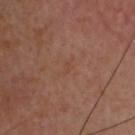- size: ~2 mm (longest diameter)
- anatomic site: the head or neck
- patient: male, aged 63–67
- lighting: cross-polarized
- imaging modality: ~15 mm tile from a whole-body skin photo
- automated lesion analysis: a footprint of about 1.5 mm², an eccentricity of roughly 0.85, and two-axis asymmetry of about 0.4; about 4 CIELAB-L* units darker than the surrounding skin and a lesion-to-skin contrast of about 4 (normalized; higher = more distinct); a border-irregularity rating of about 3.5/10